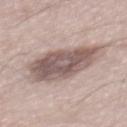Q: How was this image acquired?
A: 15 mm crop, total-body photography
Q: Patient demographics?
A: male, aged around 55